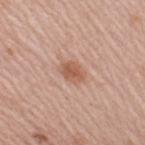biopsy status: total-body-photography surveillance lesion; no biopsy
tile lighting: white-light illumination
acquisition: 15 mm crop, total-body photography
subject: female, aged approximately 60
site: the arm
lesion size: about 3 mm